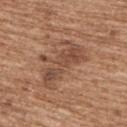Impression:
This lesion was catalogued during total-body skin photography and was not selected for biopsy.
Image and clinical context:
Captured under white-light illumination. Measured at roughly 6.5 mm in maximum diameter. A female subject, about 70 years old. On the upper back. The lesion-visualizer software estimated a lesion area of about 14 mm² and an outline eccentricity of about 0.9 (0 = round, 1 = elongated). It also reported an average lesion color of about L≈48 a*≈21 b*≈29 (CIELAB) and roughly 9 lightness units darker than nearby skin. It also reported a border-irregularity index near 6/10 and a color-variation rating of about 4.5/10. And it measured a nevus-likeness score of about 20/100. A 15 mm close-up extracted from a 3D total-body photography capture.